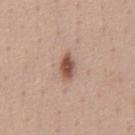workup: total-body-photography surveillance lesion; no biopsy | imaging modality: 15 mm crop, total-body photography | patient: male, in their mid- to late 40s | diameter: about 3.5 mm | lighting: white-light | location: the abdomen.